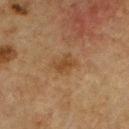workup: no biopsy performed (imaged during a skin exam); subject: male, aged 73 to 77; automated lesion analysis: a lesion color around L≈36 a*≈16 b*≈30 in CIELAB, about 6 CIELAB-L* units darker than the surrounding skin, and a normalized lesion–skin contrast near 6.5; image: total-body-photography crop, ~15 mm field of view; anatomic site: the front of the torso.